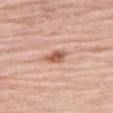{
  "biopsy_status": "not biopsied; imaged during a skin examination",
  "patient": {
    "sex": "male",
    "age_approx": 80
  },
  "image": {
    "source": "total-body photography crop",
    "field_of_view_mm": 15
  },
  "site": "right thigh",
  "lighting": "white-light"
}